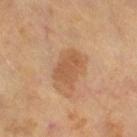| field | value |
|---|---|
| notes | no biopsy performed (imaged during a skin exam) |
| location | the left leg |
| lesion diameter | about 4 mm |
| subject | male, aged 63 to 67 |
| imaging modality | total-body-photography crop, ~15 mm field of view |
| lighting | cross-polarized illumination |